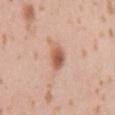Assessment:
No biopsy was performed on this lesion — it was imaged during a full skin examination and was not determined to be concerning.
Clinical summary:
The lesion is located on the left upper arm. A female patient, about 20 years old. A lesion tile, about 15 mm wide, cut from a 3D total-body photograph.